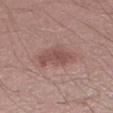Located on the left lower leg. A region of skin cropped from a whole-body photographic capture, roughly 15 mm wide. Longest diameter approximately 5 mm. The patient is a male aged approximately 40.